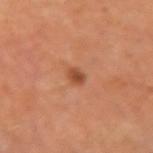patient=male, aged around 55 | automated metrics=a footprint of about 3 mm² and two-axis asymmetry of about 0.25; border irregularity of about 2 on a 0–10 scale, a within-lesion color-variation index near 1/10, and peripheral color asymmetry of about 0.5; an automated nevus-likeness rating near 90 out of 100 and lesion-presence confidence of about 100/100 | lighting=cross-polarized | lesion size=about 2.5 mm | site=the right upper arm | image source=~15 mm crop, total-body skin-cancer survey.